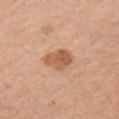Notes:
- follow-up — total-body-photography surveillance lesion; no biopsy
- location — the left upper arm
- patient — female, roughly 50 years of age
- acquisition — ~15 mm tile from a whole-body skin photo